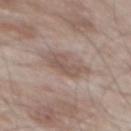Notes:
• size: ≈4 mm
• subject: male, aged 53–57
• acquisition: total-body-photography crop, ~15 mm field of view
• site: the mid back
• illumination: white-light